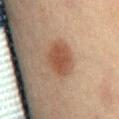  biopsy_status: not biopsied; imaged during a skin examination
  image:
    source: total-body photography crop
    field_of_view_mm: 15
  lighting: cross-polarized
  lesion_size:
    long_diameter_mm_approx: 4.5
  automated_metrics:
    nevus_likeness_0_100: 100
    lesion_detection_confidence_0_100: 100
  patient:
    sex: male
    age_approx: 65
  site: lower back